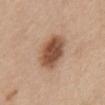The tile uses white-light illumination. A region of skin cropped from a whole-body photographic capture, roughly 15 mm wide. The lesion is located on the abdomen. A female patient aged approximately 65. Measured at roughly 5 mm in maximum diameter.